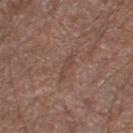Clinical impression:
Imaged during a routine full-body skin examination; the lesion was not biopsied and no histopathology is available.
Background:
Captured under white-light illumination. A male patient aged 68–72. On the right lower leg. Cropped from a whole-body photographic skin survey; the tile spans about 15 mm.